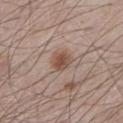biopsy_status: not biopsied; imaged during a skin examination
lesion_size:
  long_diameter_mm_approx: 2.5
patient:
  sex: male
  age_approx: 60
lighting: white-light
automated_metrics:
  vs_skin_darker_L: 10.0
  nevus_likeness_0_100: 95
  lesion_detection_confidence_0_100: 100
site: left lower leg
image:
  source: total-body photography crop
  field_of_view_mm: 15